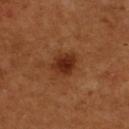An algorithmic analysis of the crop reported an area of roughly 7 mm², an eccentricity of roughly 0.65, and a shape-asymmetry score of about 0.15 (0 = symmetric). The software also gave an automated nevus-likeness rating near 95 out of 100. On the upper back. A region of skin cropped from a whole-body photographic capture, roughly 15 mm wide. Captured under cross-polarized illumination. The subject is a female aged 58 to 62.